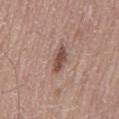• follow-up · imaged on a skin check; not biopsied
• tile lighting · white-light
• patient · male, aged around 55
• image · ~15 mm tile from a whole-body skin photo
• TBP lesion metrics · a footprint of about 5.5 mm² and two-axis asymmetry of about 0.25; an average lesion color of about L≈49 a*≈19 b*≈24 (CIELAB), roughly 12 lightness units darker than nearby skin, and a normalized border contrast of about 8.5
• diameter · ≈3.5 mm